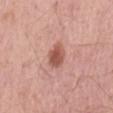No biopsy was performed on this lesion — it was imaged during a full skin examination and was not determined to be concerning. A close-up tile cropped from a whole-body skin photograph, about 15 mm across. The lesion is on the mid back. A male subject roughly 55 years of age. Captured under white-light illumination. The lesion-visualizer software estimated a lesion area of about 6 mm² and a symmetry-axis asymmetry near 0.15. It also reported a border-irregularity index near 1.5/10, internal color variation of about 2.5 on a 0–10 scale, and peripheral color asymmetry of about 1. It also reported a nevus-likeness score of about 90/100 and lesion-presence confidence of about 100/100.